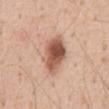No biopsy was performed on this lesion — it was imaged during a full skin examination and was not determined to be concerning.
The lesion is located on the abdomen.
A close-up tile cropped from a whole-body skin photograph, about 15 mm across.
About 5.5 mm across.
Imaged with white-light lighting.
A male patient, in their mid- to late 50s.